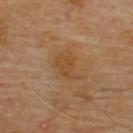Case summary:
- follow-up — catalogued during a skin exam; not biopsied
- acquisition — 15 mm crop, total-body photography
- body site — the upper back
- patient — male, aged 68–72
- illumination — cross-polarized illumination
- lesion diameter — about 4 mm